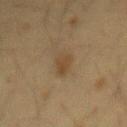Imaged during a routine full-body skin examination; the lesion was not biopsied and no histopathology is available. The tile uses cross-polarized illumination. An algorithmic analysis of the crop reported a mean CIELAB color near L≈36 a*≈11 b*≈27, roughly 6 lightness units darker than nearby skin, and a normalized border contrast of about 6. From the mid back. A female subject about 40 years old. Cropped from a total-body skin-imaging series; the visible field is about 15 mm. Longest diameter approximately 4 mm.